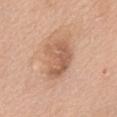The lesion was tiled from a total-body skin photograph and was not biopsied.
Located on the abdomen.
A female subject in their 60s.
A roughly 15 mm field-of-view crop from a total-body skin photograph.